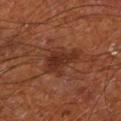The lesion was tiled from a total-body skin photograph and was not biopsied.
The subject is a male approximately 65 years of age.
Imaged with cross-polarized lighting.
From the left lower leg.
Automated tile analysis of the lesion measured two-axis asymmetry of about 0.3. The analysis additionally found an average lesion color of about L≈30 a*≈23 b*≈28 (CIELAB) and a lesion-to-skin contrast of about 7.5 (normalized; higher = more distinct). The software also gave border irregularity of about 4 on a 0–10 scale, a within-lesion color-variation index near 2.5/10, and peripheral color asymmetry of about 1. The software also gave a nevus-likeness score of about 5/100 and lesion-presence confidence of about 100/100.
The recorded lesion diameter is about 5 mm.
A 15 mm close-up tile from a total-body photography series done for melanoma screening.